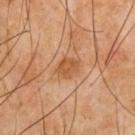biopsy status: imaged on a skin check; not biopsied | imaging modality: total-body-photography crop, ~15 mm field of view | automated lesion analysis: a shape eccentricity near 0.55 and a symmetry-axis asymmetry near 0.2; an average lesion color of about L≈51 a*≈23 b*≈37 (CIELAB), a lesion–skin lightness drop of about 9, and a normalized border contrast of about 7 | anatomic site: the chest | patient: male, aged 63–67.